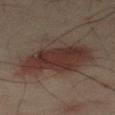This lesion was catalogued during total-body skin photography and was not selected for biopsy. The lesion is located on the mid back. The total-body-photography lesion software estimated a border-irregularity index near 4/10, internal color variation of about 5.5 on a 0–10 scale, and peripheral color asymmetry of about 1.5. A 15 mm close-up tile from a total-body photography series done for melanoma screening. Captured under cross-polarized illumination. A male subject, aged 48–52. The lesion's longest dimension is about 9.5 mm.